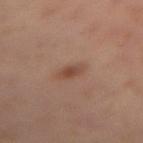The lesion was tiled from a total-body skin photograph and was not biopsied. A close-up tile cropped from a whole-body skin photograph, about 15 mm across. A female patient, in their 40s. On the right thigh.